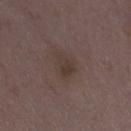This lesion was catalogued during total-body skin photography and was not selected for biopsy. A region of skin cropped from a whole-body photographic capture, roughly 15 mm wide. Automated tile analysis of the lesion measured about 5 CIELAB-L* units darker than the surrounding skin and a normalized lesion–skin contrast near 5.5. The analysis additionally found an automated nevus-likeness rating near 0 out of 100 and lesion-presence confidence of about 100/100. A female patient approximately 35 years of age. Measured at roughly 3.5 mm in maximum diameter. Captured under white-light illumination. From the right thigh.